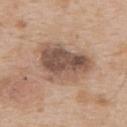Captured during whole-body skin photography for melanoma surveillance; the lesion was not biopsied.
A male subject, aged approximately 65.
An algorithmic analysis of the crop reported a lesion color around L≈52 a*≈17 b*≈26 in CIELAB, a lesion–skin lightness drop of about 14, and a lesion-to-skin contrast of about 9.5 (normalized; higher = more distinct). The software also gave a classifier nevus-likeness of about 10/100.
A close-up tile cropped from a whole-body skin photograph, about 15 mm across.
From the upper back.
Imaged with white-light lighting.